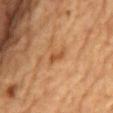This lesion was catalogued during total-body skin photography and was not selected for biopsy. On the chest. A male patient, aged approximately 85 or older. Automated tile analysis of the lesion measured an outline eccentricity of about 0.9 (0 = round, 1 = elongated) and two-axis asymmetry of about 0.35. The analysis additionally found an average lesion color of about L≈44 a*≈22 b*≈35 (CIELAB), roughly 9 lightness units darker than nearby skin, and a lesion-to-skin contrast of about 7 (normalized; higher = more distinct). Measured at roughly 2.5 mm in maximum diameter. This image is a 15 mm lesion crop taken from a total-body photograph.